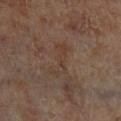Findings:
• workup — total-body-photography surveillance lesion; no biopsy
• patient — male, in their 70s
• lighting — cross-polarized
• location — the leg
• imaging modality — 15 mm crop, total-body photography
• size — ≈5 mm